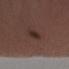workup: total-body-photography surveillance lesion; no biopsy
site: the right forearm
patient: male, in their 40s
tile lighting: white-light illumination
automated metrics: a footprint of about 3.5 mm², an outline eccentricity of about 0.8 (0 = round, 1 = elongated), and a symmetry-axis asymmetry near 0.3; about 8 CIELAB-L* units darker than the surrounding skin and a normalized lesion–skin contrast near 8; a border-irregularity index near 2.5/10, a within-lesion color-variation index near 1.5/10, and a peripheral color-asymmetry measure near 0.5; a classifier nevus-likeness of about 95/100 and a detector confidence of about 100 out of 100 that the crop contains a lesion
imaging modality: 15 mm crop, total-body photography
lesion diameter: about 2.5 mm